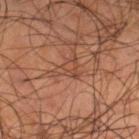Recorded during total-body skin imaging; not selected for excision or biopsy.
Located on the leg.
Captured under cross-polarized illumination.
The lesion's longest dimension is about 3 mm.
A male subject aged 53–57.
This image is a 15 mm lesion crop taken from a total-body photograph.